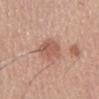biopsy status: total-body-photography surveillance lesion; no biopsy | diameter: about 4 mm | illumination: white-light | subject: male, approximately 55 years of age | site: the mid back | imaging modality: ~15 mm tile from a whole-body skin photo | image-analysis metrics: an area of roughly 8.5 mm² and an eccentricity of roughly 0.75.